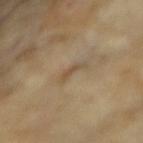Impression: This lesion was catalogued during total-body skin photography and was not selected for biopsy. Background: This is a cross-polarized tile. The total-body-photography lesion software estimated a footprint of about 2 mm². It also reported a nevus-likeness score of about 0/100. A 15 mm crop from a total-body photograph taken for skin-cancer surveillance. The lesion is located on the left forearm. A female subject in their mid- to late 70s. Measured at roughly 2.5 mm in maximum diameter.